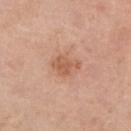Q: Was a biopsy performed?
A: total-body-photography surveillance lesion; no biopsy
Q: What did automated image analysis measure?
A: a lesion color around L≈58 a*≈24 b*≈33 in CIELAB, roughly 9 lightness units darker than nearby skin, and a normalized lesion–skin contrast near 6.5; border irregularity of about 4 on a 0–10 scale, a color-variation rating of about 2/10, and peripheral color asymmetry of about 0.5; a nevus-likeness score of about 35/100 and a detector confidence of about 100 out of 100 that the crop contains a lesion
Q: What is the anatomic site?
A: the right lower leg
Q: What is the imaging modality?
A: 15 mm crop, total-body photography
Q: Who is the patient?
A: female, aged 68–72
Q: How was the tile lit?
A: white-light illumination
Q: What is the lesion's diameter?
A: ≈3.5 mm